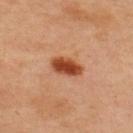Imaged during a routine full-body skin examination; the lesion was not biopsied and no histopathology is available.
The lesion is located on the upper back.
A close-up tile cropped from a whole-body skin photograph, about 15 mm across.
A male subject approximately 45 years of age.